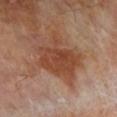Image and clinical context: A 15 mm close-up extracted from a 3D total-body photography capture. About 6 mm across. The lesion-visualizer software estimated a border-irregularity rating of about 5/10, internal color variation of about 3.5 on a 0–10 scale, and peripheral color asymmetry of about 1. And it measured a detector confidence of about 100 out of 100 that the crop contains a lesion. The lesion is on the leg. A female patient about 75 years old.